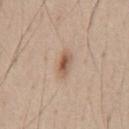Clinical impression:
The lesion was tiled from a total-body skin photograph and was not biopsied.
Background:
The lesion is on the chest. A male patient in their mid- to late 40s. Imaged with white-light lighting. Approximately 3.5 mm at its widest. A roughly 15 mm field-of-view crop from a total-body skin photograph.